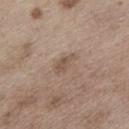A 15 mm close-up extracted from a 3D total-body photography capture.
Located on the left lower leg.
A male subject aged 68 to 72.